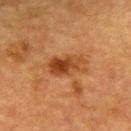Assessment:
No biopsy was performed on this lesion — it was imaged during a full skin examination and was not determined to be concerning.
Image and clinical context:
Measured at roughly 4.5 mm in maximum diameter. On the upper back. The subject is a female aged approximately 55. A close-up tile cropped from a whole-body skin photograph, about 15 mm across. Imaged with cross-polarized lighting. Automated image analysis of the tile measured a lesion area of about 8.5 mm², an eccentricity of roughly 0.85, and two-axis asymmetry of about 0.25. It also reported a mean CIELAB color near L≈36 a*≈23 b*≈34, about 10 CIELAB-L* units darker than the surrounding skin, and a lesion-to-skin contrast of about 8.5 (normalized; higher = more distinct). The analysis additionally found border irregularity of about 3.5 on a 0–10 scale, a within-lesion color-variation index near 6/10, and radial color variation of about 2.